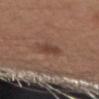Notes:
- imaging modality — 15 mm crop, total-body photography
- subject — male, roughly 55 years of age
- anatomic site — the right upper arm
- image-analysis metrics — a mean CIELAB color near L≈42 a*≈19 b*≈25; a border-irregularity index near 2/10, a within-lesion color-variation index near 2/10, and a peripheral color-asymmetry measure near 0.5; an automated nevus-likeness rating near 65 out of 100 and a detector confidence of about 100 out of 100 that the crop contains a lesion